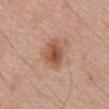Imaged during a routine full-body skin examination; the lesion was not biopsied and no histopathology is available. The lesion is on the back. A male patient aged 68–72. About 4 mm across. Captured under white-light illumination. Cropped from a whole-body photographic skin survey; the tile spans about 15 mm.